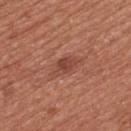workup = imaged on a skin check; not biopsied | image source = total-body-photography crop, ~15 mm field of view | body site = the upper back | subject = male, aged 63 to 67.